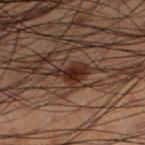follow-up — total-body-photography surveillance lesion; no biopsy | automated metrics — a footprint of about 6 mm² and a shape eccentricity near 0.8; a border-irregularity rating of about 4.5/10 | anatomic site — the left lower leg | acquisition — total-body-photography crop, ~15 mm field of view | patient — male, about 50 years old | lesion size — ≈4 mm | lighting — cross-polarized.